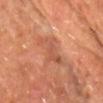Q: Was this lesion biopsied?
A: catalogued during a skin exam; not biopsied
Q: Patient demographics?
A: male, roughly 60 years of age
Q: What is the imaging modality?
A: ~15 mm crop, total-body skin-cancer survey
Q: What lighting was used for the tile?
A: cross-polarized
Q: What is the lesion's diameter?
A: ≈4.5 mm
Q: Lesion location?
A: the upper back